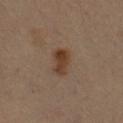Case summary:
* lighting: cross-polarized
* anatomic site: the arm
* automated lesion analysis: roughly 9 lightness units darker than nearby skin and a normalized lesion–skin contrast near 8.5; border irregularity of about 2 on a 0–10 scale and radial color variation of about 1.5
* size: about 3.5 mm
* subject: male, roughly 50 years of age
* acquisition: 15 mm crop, total-body photography